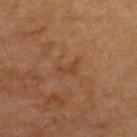Impression: Captured during whole-body skin photography for melanoma surveillance; the lesion was not biopsied.